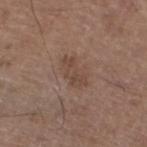Captured during whole-body skin photography for melanoma surveillance; the lesion was not biopsied. The lesion is on the left lower leg. This image is a 15 mm lesion crop taken from a total-body photograph. The patient is a male about 65 years old.